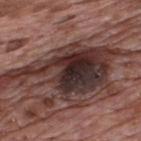notes: imaged on a skin check; not biopsied | tile lighting: white-light | patient: male, aged around 70 | location: the upper back | image source: ~15 mm tile from a whole-body skin photo | automated metrics: an area of roughly 32 mm² and an outline eccentricity of about 0.95 (0 = round, 1 = elongated); a lesion color around L≈30 a*≈18 b*≈18 in CIELAB, a lesion–skin lightness drop of about 18, and a lesion-to-skin contrast of about 15.5 (normalized; higher = more distinct); a color-variation rating of about 9/10 and a peripheral color-asymmetry measure near 2.5 | size: about 14 mm.